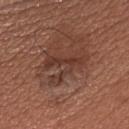Case summary:
• biopsy status · total-body-photography surveillance lesion; no biopsy
• subject · male, in their mid-30s
• body site · the chest
• imaging modality · ~15 mm tile from a whole-body skin photo